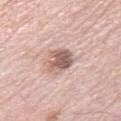Findings:
* workup — no biopsy performed (imaged during a skin exam)
* body site — the right lower leg
* size — ~3.5 mm (longest diameter)
* image source — total-body-photography crop, ~15 mm field of view
* lighting — white-light illumination
* patient — female, roughly 65 years of age